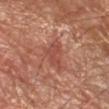Captured during whole-body skin photography for melanoma surveillance; the lesion was not biopsied. Located on the left forearm. A 15 mm close-up tile from a total-body photography series done for melanoma screening. Approximately 4.5 mm at its widest. Automated image analysis of the tile measured a mean CIELAB color near L≈49 a*≈28 b*≈28, about 8 CIELAB-L* units darker than the surrounding skin, and a normalized lesion–skin contrast near 6.